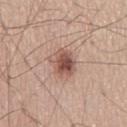Impression: Recorded during total-body skin imaging; not selected for excision or biopsy. Context: The lesion-visualizer software estimated an area of roughly 8 mm², an eccentricity of roughly 0.6, and a shape-asymmetry score of about 0.2 (0 = symmetric). The analysis additionally found an average lesion color of about L≈53 a*≈21 b*≈26 (CIELAB), a lesion–skin lightness drop of about 13, and a lesion-to-skin contrast of about 9 (normalized; higher = more distinct). Captured under white-light illumination. From the mid back. A 15 mm crop from a total-body photograph taken for skin-cancer surveillance. Approximately 3.5 mm at its widest. The subject is a male aged 58 to 62.